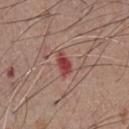notes = catalogued during a skin exam; not biopsied
patient = male, aged around 55
image source = total-body-photography crop, ~15 mm field of view
tile lighting = white-light illumination
automated metrics = a lesion color around L≈44 a*≈31 b*≈24 in CIELAB and about 12 CIELAB-L* units darker than the surrounding skin; an automated nevus-likeness rating near 0 out of 100 and lesion-presence confidence of about 100/100
size = about 3 mm
site = the chest